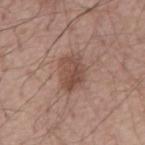<lesion>
<biopsy_status>not biopsied; imaged during a skin examination</biopsy_status>
<patient>
  <sex>male</sex>
  <age_approx>55</age_approx>
</patient>
<site>mid back</site>
<image>
  <source>total-body photography crop</source>
  <field_of_view_mm>15</field_of_view_mm>
</image>
</lesion>